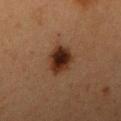Clinical impression:
The lesion was photographed on a routine skin check and not biopsied; there is no pathology result.
Background:
The lesion is located on the right upper arm. The tile uses cross-polarized illumination. The subject is a female roughly 40 years of age. The lesion's longest dimension is about 4 mm. Cropped from a total-body skin-imaging series; the visible field is about 15 mm. Automated image analysis of the tile measured a footprint of about 9.5 mm², a shape eccentricity near 0.6, and a shape-asymmetry score of about 0.15 (0 = symmetric). And it measured an average lesion color of about L≈25 a*≈18 b*≈24 (CIELAB) and a lesion–skin lightness drop of about 13. The analysis additionally found a border-irregularity rating of about 2/10 and internal color variation of about 6 on a 0–10 scale.